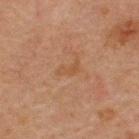Impression:
The lesion was tiled from a total-body skin photograph and was not biopsied.
Background:
A lesion tile, about 15 mm wide, cut from a 3D total-body photograph. On the upper back. The subject is a male aged around 60.